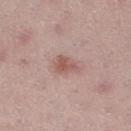Assessment: Recorded during total-body skin imaging; not selected for excision or biopsy. Image and clinical context: A lesion tile, about 15 mm wide, cut from a 3D total-body photograph. This is a white-light tile. A female patient aged 23–27. Located on the right thigh. The lesion's longest dimension is about 3 mm.